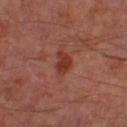Imaged during a routine full-body skin examination; the lesion was not biopsied and no histopathology is available. The total-body-photography lesion software estimated a lesion color around L≈34 a*≈28 b*≈28 in CIELAB. And it measured a border-irregularity index near 4/10. The lesion is on the right thigh. A male subject aged around 65. A region of skin cropped from a whole-body photographic capture, roughly 15 mm wide.